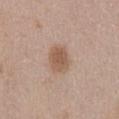The lesion was photographed on a routine skin check and not biopsied; there is no pathology result.
This is a white-light tile.
A male patient, aged approximately 50.
Cropped from a total-body skin-imaging series; the visible field is about 15 mm.
Located on the abdomen.
The lesion's longest dimension is about 3.5 mm.
The total-body-photography lesion software estimated a footprint of about 8 mm², an outline eccentricity of about 0.65 (0 = round, 1 = elongated), and a shape-asymmetry score of about 0.15 (0 = symmetric). The analysis additionally found a mean CIELAB color near L≈56 a*≈17 b*≈29, a lesion–skin lightness drop of about 10, and a lesion-to-skin contrast of about 7.5 (normalized; higher = more distinct). The analysis additionally found an automated nevus-likeness rating near 90 out of 100.